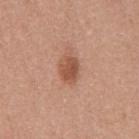  biopsy_status: not biopsied; imaged during a skin examination
  lighting: white-light
  patient:
    sex: male
    age_approx: 45
  site: back
  image:
    source: total-body photography crop
    field_of_view_mm: 15
  lesion_size:
    long_diameter_mm_approx: 3.0